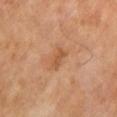| field | value |
|---|---|
| follow-up | imaged on a skin check; not biopsied |
| lesion size | ~2.5 mm (longest diameter) |
| patient | male, in their mid-60s |
| lighting | cross-polarized |
| image source | ~15 mm tile from a whole-body skin photo |
| location | the leg |